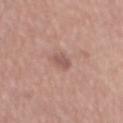The lesion was tiled from a total-body skin photograph and was not biopsied.
Captured under white-light illumination.
Approximately 2.5 mm at its widest.
The lesion is located on the back.
A male patient, about 65 years old.
A close-up tile cropped from a whole-body skin photograph, about 15 mm across.